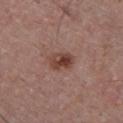The lesion was tiled from a total-body skin photograph and was not biopsied.
A male subject, aged approximately 40.
The lesion is on the chest.
A 15 mm close-up tile from a total-body photography series done for melanoma screening.